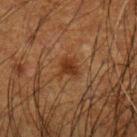Q: Was this lesion biopsied?
A: imaged on a skin check; not biopsied
Q: What did automated image analysis measure?
A: a footprint of about 5 mm² and an outline eccentricity of about 0.35 (0 = round, 1 = elongated); a lesion color around L≈27 a*≈18 b*≈28 in CIELAB, roughly 7 lightness units darker than nearby skin, and a normalized lesion–skin contrast near 8; a nevus-likeness score of about 80/100 and a lesion-detection confidence of about 100/100
Q: What are the patient's age and sex?
A: male, in their 50s
Q: How was the tile lit?
A: cross-polarized
Q: What is the lesion's diameter?
A: ≈2.5 mm
Q: Where on the body is the lesion?
A: the left forearm
Q: What is the imaging modality?
A: total-body-photography crop, ~15 mm field of view